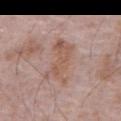This lesion was catalogued during total-body skin photography and was not selected for biopsy. Imaged with white-light lighting. The patient is a male approximately 70 years of age. The lesion is located on the abdomen. About 6 mm across. This image is a 15 mm lesion crop taken from a total-body photograph.